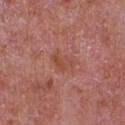<tbp_lesion>
  <biopsy_status>not biopsied; imaged during a skin examination</biopsy_status>
  <lighting>white-light</lighting>
  <patient>
    <sex>male</sex>
    <age_approx>65</age_approx>
  </patient>
  <lesion_size>
    <long_diameter_mm_approx>3.0</long_diameter_mm_approx>
  </lesion_size>
  <site>chest</site>
  <automated_metrics>
    <area_mm2_approx>4.5</area_mm2_approx>
    <eccentricity>0.75</eccentricity>
    <shape_asymmetry>0.45</shape_asymmetry>
    <cielab_L>48</cielab_L>
    <cielab_a>26</cielab_a>
    <cielab_b>29</cielab_b>
    <vs_skin_darker_L>6.0</vs_skin_darker_L>
    <vs_skin_contrast_norm>6.0</vs_skin_contrast_norm>
    <color_variation_0_10>3.0</color_variation_0_10>
    <peripheral_color_asymmetry>1.0</peripheral_color_asymmetry>
  </automated_metrics>
  <image>
    <source>total-body photography crop</source>
    <field_of_view_mm>15</field_of_view_mm>
  </image>
</tbp_lesion>